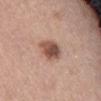Case summary:
* follow-up · catalogued during a skin exam; not biopsied